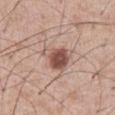Q: Was a biopsy performed?
A: imaged on a skin check; not biopsied
Q: What lighting was used for the tile?
A: white-light illumination
Q: What is the anatomic site?
A: the abdomen
Q: What are the patient's age and sex?
A: male, aged 53 to 57
Q: Lesion size?
A: about 4 mm
Q: What kind of image is this?
A: ~15 mm tile from a whole-body skin photo